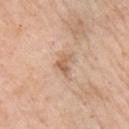notes — total-body-photography surveillance lesion; no biopsy | body site — the right upper arm | lesion diameter — ~2.5 mm (longest diameter) | acquisition — ~15 mm crop, total-body skin-cancer survey | subject — female, aged approximately 65 | automated metrics — an area of roughly 3.5 mm², an outline eccentricity of about 0.8 (0 = round, 1 = elongated), and a symmetry-axis asymmetry near 0.5; a mean CIELAB color near L≈61 a*≈20 b*≈33, about 10 CIELAB-L* units darker than the surrounding skin, and a normalized lesion–skin contrast near 7; border irregularity of about 5 on a 0–10 scale, internal color variation of about 0.5 on a 0–10 scale, and peripheral color asymmetry of about 0 | tile lighting — white-light illumination.